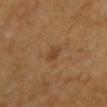<record>
<biopsy_status>not biopsied; imaged during a skin examination</biopsy_status>
<site>upper back</site>
<patient>
  <sex>female</sex>
  <age_approx>55</age_approx>
</patient>
<image>
  <source>total-body photography crop</source>
  <field_of_view_mm>15</field_of_view_mm>
</image>
</record>